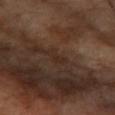Impression: This lesion was catalogued during total-body skin photography and was not selected for biopsy. Context: The subject is a female aged around 70. Imaged with cross-polarized lighting. The lesion is located on the left forearm. The total-body-photography lesion software estimated an average lesion color of about L≈27 a*≈16 b*≈23 (CIELAB), roughly 4 lightness units darker than nearby skin, and a normalized border contrast of about 5. And it measured border irregularity of about 4.5 on a 0–10 scale, a color-variation rating of about 0/10, and peripheral color asymmetry of about 0. The software also gave a nevus-likeness score of about 0/100 and a lesion-detection confidence of about 60/100. Approximately 3 mm at its widest. A close-up tile cropped from a whole-body skin photograph, about 15 mm across.